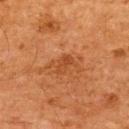| feature | finding |
|---|---|
| subject | male, approximately 65 years of age |
| imaging modality | 15 mm crop, total-body photography |
| illumination | cross-polarized |
| anatomic site | the upper back |